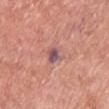{
  "biopsy_status": "not biopsied; imaged during a skin examination",
  "lesion_size": {
    "long_diameter_mm_approx": 3.0
  },
  "automated_metrics": {
    "eccentricity": 0.7,
    "cielab_L": 55,
    "cielab_a": 23,
    "cielab_b": 20,
    "vs_skin_contrast_norm": 8.5,
    "border_irregularity_0_10": 4.0,
    "color_variation_0_10": 7.5,
    "peripheral_color_asymmetry": 2.0,
    "nevus_likeness_0_100": 0,
    "lesion_detection_confidence_0_100": 100
  },
  "lighting": "white-light",
  "patient": {
    "sex": "male",
    "age_approx": 60
  },
  "site": "chest",
  "image": {
    "source": "total-body photography crop",
    "field_of_view_mm": 15
  }
}